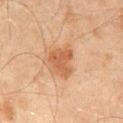– workup · no biopsy performed (imaged during a skin exam)